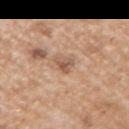| key | value |
|---|---|
| biopsy status | imaged on a skin check; not biopsied |
| body site | the right upper arm |
| subject | male, aged around 65 |
| acquisition | ~15 mm crop, total-body skin-cancer survey |